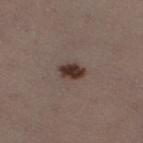workup = imaged on a skin check; not biopsied | tile lighting = white-light | imaging modality = 15 mm crop, total-body photography | lesion diameter = about 3 mm | location = the leg | subject = female, aged around 30.